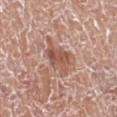Impression: Captured during whole-body skin photography for melanoma surveillance; the lesion was not biopsied. Background: The patient is a male roughly 75 years of age. On the right lower leg. This is a white-light tile. Cropped from a whole-body photographic skin survey; the tile spans about 15 mm. Measured at roughly 4.5 mm in maximum diameter.